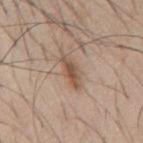The lesion was photographed on a routine skin check and not biopsied; there is no pathology result. A roughly 15 mm field-of-view crop from a total-body skin photograph. Imaged with white-light lighting. Located on the back. The lesion's longest dimension is about 4 mm. A male subject aged approximately 45.